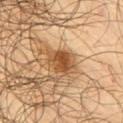automated lesion analysis: an eccentricity of roughly 0.55 and a shape-asymmetry score of about 0.2 (0 = symmetric); a mean CIELAB color near L≈49 a*≈20 b*≈37, a lesion–skin lightness drop of about 13, and a lesion-to-skin contrast of about 9.5 (normalized; higher = more distinct); peripheral color asymmetry of about 1.5
lighting: cross-polarized
acquisition: total-body-photography crop, ~15 mm field of view
subject: male, aged around 65
location: the right upper arm